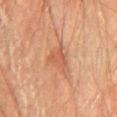The lesion was tiled from a total-body skin photograph and was not biopsied.
Cropped from a total-body skin-imaging series; the visible field is about 15 mm.
The lesion is on the chest.
A male subject in their mid-70s.